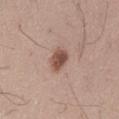Clinical impression: Part of a total-body skin-imaging series; this lesion was reviewed on a skin check and was not flagged for biopsy. Background: Captured under white-light illumination. The lesion is located on the left thigh. The recorded lesion diameter is about 3 mm. A region of skin cropped from a whole-body photographic capture, roughly 15 mm wide. A male patient roughly 45 years of age. The lesion-visualizer software estimated an area of roughly 5.5 mm² and a shape eccentricity near 0.65. It also reported an average lesion color of about L≈51 a*≈20 b*≈25 (CIELAB) and roughly 13 lightness units darker than nearby skin. The software also gave a border-irregularity rating of about 1.5/10, a color-variation rating of about 4/10, and a peripheral color-asymmetry measure near 1.5.